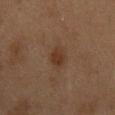Recorded during total-body skin imaging; not selected for excision or biopsy. A female subject approximately 40 years of age. Approximately 3 mm at its widest. A close-up tile cropped from a whole-body skin photograph, about 15 mm across. Automated tile analysis of the lesion measured a lesion area of about 4 mm² and an eccentricity of roughly 0.8. The software also gave border irregularity of about 2 on a 0–10 scale, a color-variation rating of about 1/10, and peripheral color asymmetry of about 0.5. The tile uses cross-polarized illumination. From the mid back.